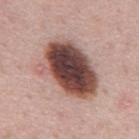  biopsy_status: not biopsied; imaged during a skin examination
  lighting: white-light
  site: mid back
  patient:
    sex: male
    age_approx: 50
  lesion_size:
    long_diameter_mm_approx: 8.0
  automated_metrics:
    cielab_L: 45
    cielab_a: 20
    cielab_b: 22
    vs_skin_darker_L: 22.0
    vs_skin_contrast_norm: 15.0
    lesion_detection_confidence_0_100: 100
  image:
    source: total-body photography crop
    field_of_view_mm: 15